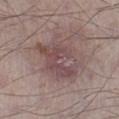| key | value |
|---|---|
| notes | no biopsy performed (imaged during a skin exam) |